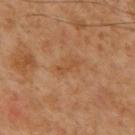Captured during whole-body skin photography for melanoma surveillance; the lesion was not biopsied. A male patient, aged approximately 60. Imaged with cross-polarized lighting. A lesion tile, about 15 mm wide, cut from a 3D total-body photograph. On the mid back.